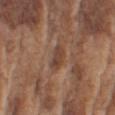The lesion was photographed on a routine skin check and not biopsied; there is no pathology result. Located on the right upper arm. Cropped from a total-body skin-imaging series; the visible field is about 15 mm. A male subject aged around 75. The lesion-visualizer software estimated an average lesion color of about L≈42 a*≈20 b*≈28 (CIELAB), about 8 CIELAB-L* units darker than the surrounding skin, and a normalized lesion–skin contrast near 6.5.